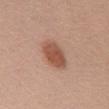Clinical impression:
Recorded during total-body skin imaging; not selected for excision or biopsy.
Clinical summary:
A female subject, approximately 60 years of age. The lesion is located on the mid back. This is a white-light tile. A roughly 15 mm field-of-view crop from a total-body skin photograph. Measured at roughly 4.5 mm in maximum diameter.